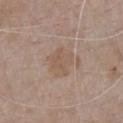Located on the chest. The subject is a male in their mid- to late 70s. A 15 mm crop from a total-body photograph taken for skin-cancer surveillance. Captured under white-light illumination.